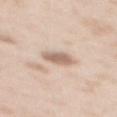notes = no biopsy performed (imaged during a skin exam)
image source = ~15 mm tile from a whole-body skin photo
TBP lesion metrics = a lesion area of about 5.5 mm² and a symmetry-axis asymmetry near 0.2; a mean CIELAB color near L≈64 a*≈16 b*≈27 and a normalized lesion–skin contrast near 8; border irregularity of about 2 on a 0–10 scale, a within-lesion color-variation index near 3/10, and peripheral color asymmetry of about 1; a nevus-likeness score of about 55/100 and a lesion-detection confidence of about 100/100
location = the mid back
size = ~3 mm (longest diameter)
subject = female, about 50 years old
tile lighting = white-light